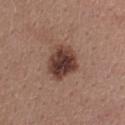| field | value |
|---|---|
| notes | imaged on a skin check; not biopsied |
| TBP lesion metrics | a border-irregularity rating of about 2/10, a within-lesion color-variation index near 6/10, and a peripheral color-asymmetry measure near 2; a detector confidence of about 100 out of 100 that the crop contains a lesion |
| illumination | white-light illumination |
| image source | total-body-photography crop, ~15 mm field of view |
| patient | female, aged around 40 |
| body site | the upper back |
| size | ≈4.5 mm |